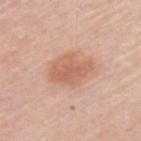Part of a total-body skin-imaging series; this lesion was reviewed on a skin check and was not flagged for biopsy. A 15 mm close-up extracted from a 3D total-body photography capture. About 5.5 mm across. Captured under white-light illumination. From the upper back. The patient is a male about 60 years old.